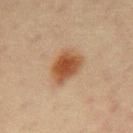Clinical impression: Part of a total-body skin-imaging series; this lesion was reviewed on a skin check and was not flagged for biopsy. Acquisition and patient details: A male patient aged approximately 70. A 15 mm close-up extracted from a 3D total-body photography capture. The lesion is located on the abdomen. Approximately 4.5 mm at its widest.